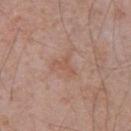Case summary:
* notes · imaged on a skin check; not biopsied
* subject · male, about 70 years old
* imaging modality · 15 mm crop, total-body photography
* automated metrics · a lesion area of about 3 mm², an eccentricity of roughly 0.75, and two-axis asymmetry of about 0.7; a lesion color around L≈54 a*≈20 b*≈29 in CIELAB, roughly 6 lightness units darker than nearby skin, and a normalized lesion–skin contrast near 5
* location · the chest
* illumination · white-light illumination
* diameter · about 3 mm